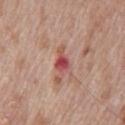Part of a total-body skin-imaging series; this lesion was reviewed on a skin check and was not flagged for biopsy.
Measured at roughly 3 mm in maximum diameter.
Automated image analysis of the tile measured a footprint of about 4 mm² and an eccentricity of roughly 0.8. And it measured a lesion color around L≈51 a*≈31 b*≈25 in CIELAB, about 12 CIELAB-L* units darker than the surrounding skin, and a lesion-to-skin contrast of about 8.5 (normalized; higher = more distinct). It also reported a border-irregularity index near 3.5/10 and peripheral color asymmetry of about 1. The analysis additionally found an automated nevus-likeness rating near 0 out of 100 and a lesion-detection confidence of about 100/100.
A lesion tile, about 15 mm wide, cut from a 3D total-body photograph.
A male subject, aged 68–72.
From the chest.